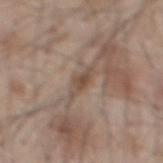<record>
  <biopsy_status>not biopsied; imaged during a skin examination</biopsy_status>
  <image>
    <source>total-body photography crop</source>
    <field_of_view_mm>15</field_of_view_mm>
  </image>
  <site>mid back</site>
  <automated_metrics>
    <area_mm2_approx>3.5</area_mm2_approx>
    <eccentricity>0.9</eccentricity>
    <shape_asymmetry>0.45</shape_asymmetry>
    <cielab_L>48</cielab_L>
    <cielab_a>14</cielab_a>
    <cielab_b>25</cielab_b>
    <vs_skin_darker_L>9.0</vs_skin_darker_L>
    <vs_skin_contrast_norm>7.0</vs_skin_contrast_norm>
    <border_irregularity_0_10>5.0</border_irregularity_0_10>
    <color_variation_0_10>2.5</color_variation_0_10>
    <peripheral_color_asymmetry>1.0</peripheral_color_asymmetry>
  </automated_metrics>
  <lighting>white-light</lighting>
  <patient>
    <sex>male</sex>
    <age_approx>45</age_approx>
  </patient>
</record>